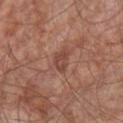Imaged during a routine full-body skin examination; the lesion was not biopsied and no histopathology is available. A close-up tile cropped from a whole-body skin photograph, about 15 mm across. Longest diameter approximately 3 mm. On the chest. The patient is a male aged approximately 65.